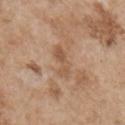biopsy status: no biopsy performed (imaged during a skin exam) | lesion diameter: ~4 mm (longest diameter) | anatomic site: the chest | image: ~15 mm tile from a whole-body skin photo | patient: male, aged 53–57 | illumination: white-light illumination.